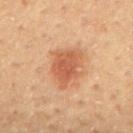Clinical impression:
Part of a total-body skin-imaging series; this lesion was reviewed on a skin check and was not flagged for biopsy.
Clinical summary:
A male subject, in their mid- to late 50s. A 15 mm close-up extracted from a 3D total-body photography capture. Longest diameter approximately 4.5 mm. Located on the mid back.